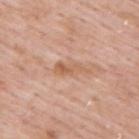Captured during whole-body skin photography for melanoma surveillance; the lesion was not biopsied. A lesion tile, about 15 mm wide, cut from a 3D total-body photograph. A male subject roughly 55 years of age. This is a white-light tile. The lesion is on the upper back. Approximately 4 mm at its widest.